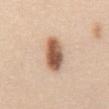| field | value |
|---|---|
| notes | catalogued during a skin exam; not biopsied |
| image source | ~15 mm tile from a whole-body skin photo |
| location | the abdomen |
| subject | female, roughly 40 years of age |